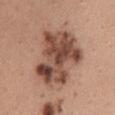Part of a total-body skin-imaging series; this lesion was reviewed on a skin check and was not flagged for biopsy. A female patient, aged around 35. An algorithmic analysis of the crop reported an area of roughly 29 mm², a shape eccentricity near 0.75, and two-axis asymmetry of about 0.35. It also reported a lesion color around L≈47 a*≈21 b*≈27 in CIELAB, about 16 CIELAB-L* units darker than the surrounding skin, and a lesion-to-skin contrast of about 11 (normalized; higher = more distinct). And it measured border irregularity of about 4.5 on a 0–10 scale, a within-lesion color-variation index near 8.5/10, and a peripheral color-asymmetry measure near 3.5. This is a white-light tile. This image is a 15 mm lesion crop taken from a total-body photograph. On the mid back.